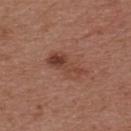notes = imaged on a skin check; not biopsied | acquisition = ~15 mm tile from a whole-body skin photo | patient = male, in their mid- to late 50s | location = the front of the torso.